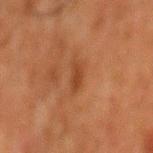biopsy status: no biopsy performed (imaged during a skin exam)
lesion size: ~3 mm (longest diameter)
acquisition: ~15 mm crop, total-body skin-cancer survey
tile lighting: cross-polarized illumination
automated lesion analysis: an average lesion color of about L≈34 a*≈21 b*≈31 (CIELAB) and a normalized border contrast of about 6.5
subject: male, aged around 80
site: the mid back